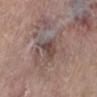biopsy status=no biopsy performed (imaged during a skin exam).